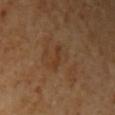Q: Is there a histopathology result?
A: total-body-photography surveillance lesion; no biopsy
Q: What is the imaging modality?
A: ~15 mm tile from a whole-body skin photo
Q: Automated lesion metrics?
A: a lesion area of about 2.5 mm², an eccentricity of roughly 0.9, and a symmetry-axis asymmetry near 0.3; a border-irregularity index near 3.5/10, a color-variation rating of about 0/10, and radial color variation of about 0; a nevus-likeness score of about 0/100 and a detector confidence of about 100 out of 100 that the crop contains a lesion
Q: Lesion location?
A: the right upper arm
Q: Lesion size?
A: ~2.5 mm (longest diameter)
Q: Illumination type?
A: cross-polarized
Q: What are the patient's age and sex?
A: female